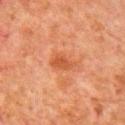Q: Was this lesion biopsied?
A: catalogued during a skin exam; not biopsied
Q: Lesion location?
A: the right upper arm
Q: What did automated image analysis measure?
A: a lesion color around L≈43 a*≈25 b*≈34 in CIELAB, a lesion–skin lightness drop of about 8, and a normalized lesion–skin contrast near 7; an automated nevus-likeness rating near 0 out of 100 and a detector confidence of about 100 out of 100 that the crop contains a lesion
Q: What kind of image is this?
A: ~15 mm crop, total-body skin-cancer survey
Q: Patient demographics?
A: male, aged approximately 80